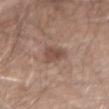This lesion was catalogued during total-body skin photography and was not selected for biopsy. The subject is a male approximately 75 years of age. Automated tile analysis of the lesion measured a footprint of about 5.5 mm², an eccentricity of roughly 0.8, and a shape-asymmetry score of about 0.35 (0 = symmetric). It also reported a within-lesion color-variation index near 2/10. This is a white-light tile. A region of skin cropped from a whole-body photographic capture, roughly 15 mm wide. Measured at roughly 3.5 mm in maximum diameter. The lesion is on the abdomen.